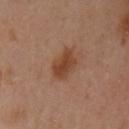Clinical impression: The lesion was photographed on a routine skin check and not biopsied; there is no pathology result. Context: Cropped from a total-body skin-imaging series; the visible field is about 15 mm. From the left upper arm. A female subject in their 40s. The lesion's longest dimension is about 3.5 mm.